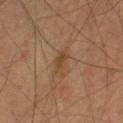| key | value |
|---|---|
| workup | catalogued during a skin exam; not biopsied |
| anatomic site | the left thigh |
| image | 15 mm crop, total-body photography |
| illumination | cross-polarized |
| subject | male, aged approximately 60 |
| TBP lesion metrics | a lesion color around L≈39 a*≈17 b*≈29 in CIELAB, roughly 7 lightness units darker than nearby skin, and a lesion-to-skin contrast of about 6.5 (normalized; higher = more distinct); border irregularity of about 3.5 on a 0–10 scale, internal color variation of about 1 on a 0–10 scale, and a peripheral color-asymmetry measure near 0.5 |